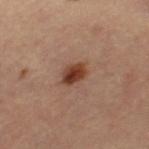Q: Was a biopsy performed?
A: catalogued during a skin exam; not biopsied
Q: Automated lesion metrics?
A: a mean CIELAB color near L≈38 a*≈23 b*≈29, a lesion–skin lightness drop of about 13, and a normalized lesion–skin contrast near 11; a border-irregularity index near 2/10 and a peripheral color-asymmetry measure near 2
Q: Who is the patient?
A: female, approximately 45 years of age
Q: Lesion size?
A: about 3 mm
Q: How was this image acquired?
A: 15 mm crop, total-body photography
Q: Lesion location?
A: the right thigh